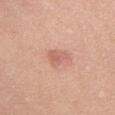* follow-up · no biopsy performed (imaged during a skin exam)
* lighting · white-light
* acquisition · ~15 mm crop, total-body skin-cancer survey
* lesion size · ~2.5 mm (longest diameter)
* image-analysis metrics · a lesion area of about 4 mm² and two-axis asymmetry of about 0.4; a border-irregularity rating of about 3.5/10 and internal color variation of about 1.5 on a 0–10 scale
* location · the chest
* patient · female, aged 38 to 42